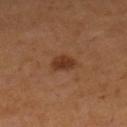Findings:
• follow-up: catalogued during a skin exam; not biopsied
• automated lesion analysis: a lesion–skin lightness drop of about 9 and a lesion-to-skin contrast of about 8 (normalized; higher = more distinct); a color-variation rating of about 2.5/10 and peripheral color asymmetry of about 1
• lesion diameter: ≈3 mm
• image: ~15 mm tile from a whole-body skin photo
• site: the right lower leg
• lighting: cross-polarized illumination
• patient: female, aged approximately 55